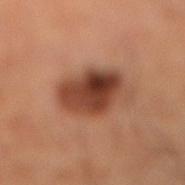Imaged during a routine full-body skin examination; the lesion was not biopsied and no histopathology is available. The lesion-visualizer software estimated a border-irregularity index near 2/10 and radial color variation of about 3. Imaged with cross-polarized lighting. A male subject aged 58–62. Approximately 5.5 mm at its widest. A close-up tile cropped from a whole-body skin photograph, about 15 mm across. The lesion is located on the right lower leg.